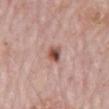Q: Was this lesion biopsied?
A: catalogued during a skin exam; not biopsied
Q: Patient demographics?
A: male, in their 80s
Q: What kind of image is this?
A: ~15 mm crop, total-body skin-cancer survey
Q: Where on the body is the lesion?
A: the back